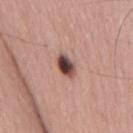The lesion was photographed on a routine skin check and not biopsied; there is no pathology result.